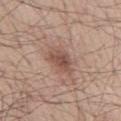Recorded during total-body skin imaging; not selected for excision or biopsy. The lesion is located on the chest. The patient is a male in their mid-50s. About 5.5 mm across. A 15 mm close-up extracted from a 3D total-body photography capture.